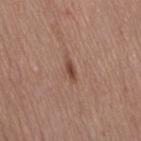Clinical impression: The lesion was tiled from a total-body skin photograph and was not biopsied. Acquisition and patient details: On the right thigh. A female subject aged approximately 40. Captured under white-light illumination. An algorithmic analysis of the crop reported an area of roughly 2.5 mm² and an eccentricity of roughly 0.9. The analysis additionally found border irregularity of about 4 on a 0–10 scale and peripheral color asymmetry of about 0. Longest diameter approximately 2.5 mm. A roughly 15 mm field-of-view crop from a total-body skin photograph.